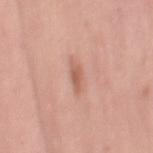workup=no biopsy performed (imaged during a skin exam)
image=15 mm crop, total-body photography
lesion size=~3 mm (longest diameter)
location=the right thigh
illumination=white-light
subject=female, aged 48 to 52